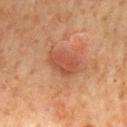Clinical impression: Recorded during total-body skin imaging; not selected for excision or biopsy. Background: On the mid back. A male patient, roughly 65 years of age. About 4 mm across. A 15 mm close-up tile from a total-body photography series done for melanoma screening. Imaged with cross-polarized lighting.